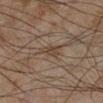Part of a total-body skin-imaging series; this lesion was reviewed on a skin check and was not flagged for biopsy.
The tile uses cross-polarized illumination.
A close-up tile cropped from a whole-body skin photograph, about 15 mm across.
The subject is a male aged 43 to 47.
The lesion-visualizer software estimated a lesion area of about 4 mm², an eccentricity of roughly 0.8, and a shape-asymmetry score of about 0.35 (0 = symmetric).
The lesion is located on the right lower leg.
Longest diameter approximately 3 mm.